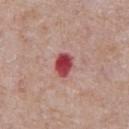follow-up: imaged on a skin check; not biopsied | image-analysis metrics: a footprint of about 5 mm² and a shape-asymmetry score of about 0.2 (0 = symmetric); lesion-presence confidence of about 100/100 | location: the front of the torso | acquisition: total-body-photography crop, ~15 mm field of view | patient: male, about 60 years old.